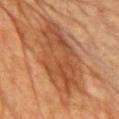Recorded during total-body skin imaging; not selected for excision or biopsy. The lesion is located on the chest. The total-body-photography lesion software estimated an area of roughly 38 mm², an eccentricity of roughly 0.9, and a shape-asymmetry score of about 0.2 (0 = symmetric). The software also gave a classifier nevus-likeness of about 30/100 and lesion-presence confidence of about 95/100. About 10.5 mm across. Cropped from a total-body skin-imaging series; the visible field is about 15 mm. Captured under cross-polarized illumination. A female subject approximately 80 years of age.